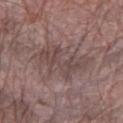biopsy status: no biopsy performed (imaged during a skin exam) | TBP lesion metrics: an area of roughly 19 mm² and a shape-asymmetry score of about 0.35 (0 = symmetric); a mean CIELAB color near L≈46 a*≈16 b*≈18, a lesion–skin lightness drop of about 7, and a normalized border contrast of about 5.5; a color-variation rating of about 4/10 and peripheral color asymmetry of about 1.5 | tile lighting: white-light | body site: the arm | size: ~6.5 mm (longest diameter) | subject: male, aged approximately 65 | image: total-body-photography crop, ~15 mm field of view.